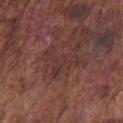notes = no biopsy performed (imaged during a skin exam); TBP lesion metrics = border irregularity of about 9.5 on a 0–10 scale, a color-variation rating of about 2/10, and a peripheral color-asymmetry measure near 0.5; subject = male, aged 73 to 77; size = about 4.5 mm; illumination = white-light illumination; image = ~15 mm tile from a whole-body skin photo; anatomic site = the front of the torso.